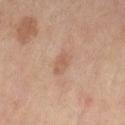Assessment:
Captured during whole-body skin photography for melanoma surveillance; the lesion was not biopsied.
Acquisition and patient details:
A 15 mm close-up extracted from a 3D total-body photography capture. The patient is a female aged 58–62. The lesion-visualizer software estimated a border-irregularity rating of about 2/10 and radial color variation of about 1. The analysis additionally found a classifier nevus-likeness of about 5/100 and a lesion-detection confidence of about 100/100. On the left thigh. Approximately 3 mm at its widest.